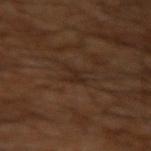follow-up: catalogued during a skin exam; not biopsied | image: 15 mm crop, total-body photography | patient: male, aged approximately 60 | anatomic site: the left arm | illumination: cross-polarized | lesion size: ≈3 mm.